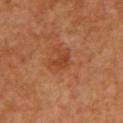workup = total-body-photography surveillance lesion; no biopsy | subject = female, in their mid-50s | site = the front of the torso | image source = 15 mm crop, total-body photography | lesion diameter = ≈2.5 mm | illumination = cross-polarized illumination | TBP lesion metrics = a mean CIELAB color near L≈44 a*≈28 b*≈38 and a normalized border contrast of about 6; a detector confidence of about 100 out of 100 that the crop contains a lesion.